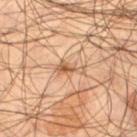| field | value |
|---|---|
| notes | catalogued during a skin exam; not biopsied |
| subject | male, in their mid- to late 50s |
| site | the left thigh |
| TBP lesion metrics | an average lesion color of about L≈55 a*≈19 b*≈33 (CIELAB), roughly 10 lightness units darker than nearby skin, and a normalized lesion–skin contrast near 7; border irregularity of about 5.5 on a 0–10 scale, a within-lesion color-variation index near 2/10, and a peripheral color-asymmetry measure near 0.5; an automated nevus-likeness rating near 30 out of 100 and a lesion-detection confidence of about 85/100 |
| imaging modality | 15 mm crop, total-body photography |